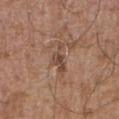Part of a total-body skin-imaging series; this lesion was reviewed on a skin check and was not flagged for biopsy. This is a white-light tile. This image is a 15 mm lesion crop taken from a total-body photograph. The recorded lesion diameter is about 4 mm. A male patient, aged approximately 75. On the abdomen.